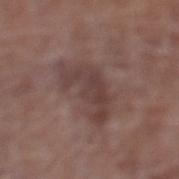Clinical impression: Captured during whole-body skin photography for melanoma surveillance; the lesion was not biopsied. Image and clinical context: The patient is a female aged 78–82. A region of skin cropped from a whole-body photographic capture, roughly 15 mm wide. The lesion's longest dimension is about 5.5 mm. The lesion is on the left lower leg.